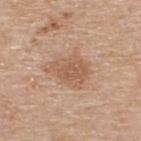Q: Was a biopsy performed?
A: no biopsy performed (imaged during a skin exam)
Q: What is the lesion's diameter?
A: about 4 mm
Q: Automated lesion metrics?
A: a footprint of about 10 mm², an outline eccentricity of about 0.7 (0 = round, 1 = elongated), and a shape-asymmetry score of about 0.3 (0 = symmetric); a mean CIELAB color near L≈57 a*≈20 b*≈31, roughly 9 lightness units darker than nearby skin, and a normalized border contrast of about 6; a border-irregularity rating of about 3.5/10, a within-lesion color-variation index near 2.5/10, and a peripheral color-asymmetry measure near 1; an automated nevus-likeness rating near 10 out of 100 and a detector confidence of about 100 out of 100 that the crop contains a lesion
Q: How was this image acquired?
A: total-body-photography crop, ~15 mm field of view
Q: Illumination type?
A: white-light
Q: Patient demographics?
A: male, in their 60s
Q: What is the anatomic site?
A: the upper back